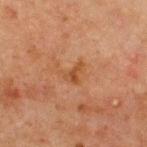Automated image analysis of the tile measured roughly 6 lightness units darker than nearby skin and a normalized lesion–skin contrast near 6. The analysis additionally found border irregularity of about 5 on a 0–10 scale, internal color variation of about 3.5 on a 0–10 scale, and a peripheral color-asymmetry measure near 1. It also reported an automated nevus-likeness rating near 0 out of 100 and a lesion-detection confidence of about 100/100.
Captured under cross-polarized illumination.
A 15 mm close-up extracted from a 3D total-body photography capture.
From the chest.
A male patient, aged 63–67.